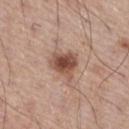The lesion was photographed on a routine skin check and not biopsied; there is no pathology result. Located on the leg. Measured at roughly 4.5 mm in maximum diameter. A male subject, aged around 60. Captured under white-light illumination. Cropped from a whole-body photographic skin survey; the tile spans about 15 mm.